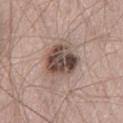– notes — catalogued during a skin exam; not biopsied
– TBP lesion metrics — a mean CIELAB color near L≈46 a*≈15 b*≈21 and a lesion-to-skin contrast of about 12 (normalized; higher = more distinct)
– lighting — white-light
– subject — male, about 60 years old
– body site — the right thigh
– acquisition — 15 mm crop, total-body photography
– size — ≈4.5 mm